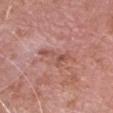Captured during whole-body skin photography for melanoma surveillance; the lesion was not biopsied.
Approximately 4.5 mm at its widest.
An algorithmic analysis of the crop reported a classifier nevus-likeness of about 0/100 and a lesion-detection confidence of about 100/100.
A male subject, about 75 years old.
The lesion is on the head or neck.
A region of skin cropped from a whole-body photographic capture, roughly 15 mm wide.